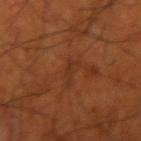Captured during whole-body skin photography for melanoma surveillance; the lesion was not biopsied. A 15 mm crop from a total-body photograph taken for skin-cancer surveillance. The total-body-photography lesion software estimated a lesion area of about 2.5 mm² and two-axis asymmetry of about 0.55. It also reported a classifier nevus-likeness of about 0/100 and a detector confidence of about 80 out of 100 that the crop contains a lesion. Located on the right upper arm. The patient is a male aged 68 to 72. Measured at roughly 3 mm in maximum diameter. Captured under cross-polarized illumination.